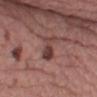From the left lower leg.
Cropped from a whole-body photographic skin survey; the tile spans about 15 mm.
The total-body-photography lesion software estimated an area of roughly 6 mm², an eccentricity of roughly 0.75, and a symmetry-axis asymmetry near 0.45. The software also gave a lesion color around L≈40 a*≈22 b*≈21 in CIELAB, about 10 CIELAB-L* units darker than the surrounding skin, and a normalized border contrast of about 8.5. The analysis additionally found border irregularity of about 5.5 on a 0–10 scale, a color-variation rating of about 4/10, and radial color variation of about 1.
A female subject, about 50 years old.
This is a white-light tile.